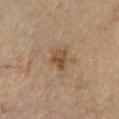No biopsy was performed on this lesion — it was imaged during a full skin examination and was not determined to be concerning.
A 15 mm close-up tile from a total-body photography series done for melanoma screening.
An algorithmic analysis of the crop reported roughly 8 lightness units darker than nearby skin and a lesion-to-skin contrast of about 7.5 (normalized; higher = more distinct). The analysis additionally found a border-irregularity index near 3.5/10. It also reported an automated nevus-likeness rating near 15 out of 100 and lesion-presence confidence of about 100/100.
Captured under cross-polarized illumination.
The lesion is located on the left lower leg.
A male patient about 65 years old.